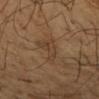No biopsy was performed on this lesion — it was imaged during a full skin examination and was not determined to be concerning.
The total-body-photography lesion software estimated a footprint of about 4.5 mm², an outline eccentricity of about 0.8 (0 = round, 1 = elongated), and two-axis asymmetry of about 0.55. The software also gave a nevus-likeness score of about 0/100 and lesion-presence confidence of about 95/100.
A region of skin cropped from a whole-body photographic capture, roughly 15 mm wide.
The lesion is located on the left forearm.
The recorded lesion diameter is about 3 mm.
Imaged with cross-polarized lighting.
A male subject, aged around 65.